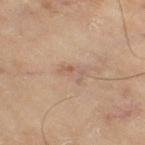The lesion was tiled from a total-body skin photograph and was not biopsied. This is a cross-polarized tile. This image is a 15 mm lesion crop taken from a total-body photograph. The patient is a male roughly 65 years of age. Approximately 2.5 mm at its widest. The lesion-visualizer software estimated an outline eccentricity of about 0.85 (0 = round, 1 = elongated). It also reported an average lesion color of about L≈58 a*≈19 b*≈28 (CIELAB), a lesion–skin lightness drop of about 7, and a normalized lesion–skin contrast near 5. The analysis additionally found a border-irregularity index near 7.5/10, a color-variation rating of about 0/10, and a peripheral color-asymmetry measure near 0. The lesion is located on the right thigh.